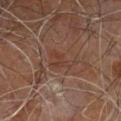Part of a total-body skin-imaging series; this lesion was reviewed on a skin check and was not flagged for biopsy. Approximately 3.5 mm at its widest. This is a cross-polarized tile. A male patient approximately 60 years of age. This image is a 15 mm lesion crop taken from a total-body photograph. Automated tile analysis of the lesion measured a lesion color around L≈36 a*≈19 b*≈25 in CIELAB, a lesion–skin lightness drop of about 5, and a normalized border contrast of about 5.5. And it measured a lesion-detection confidence of about 70/100. The lesion is on the right leg.